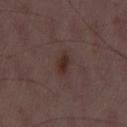follow-up=catalogued during a skin exam; not biopsied | acquisition=15 mm crop, total-body photography | subject=male, in their 50s.